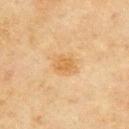Assessment: Part of a total-body skin-imaging series; this lesion was reviewed on a skin check and was not flagged for biopsy. Background: A 15 mm close-up extracted from a 3D total-body photography capture. On the upper back. Imaged with cross-polarized lighting. The patient is a female about 60 years old.